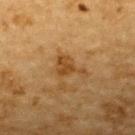The lesion was photographed on a routine skin check and not biopsied; there is no pathology result. The lesion is located on the upper back. The tile uses cross-polarized illumination. A male patient, aged approximately 85 or older. A roughly 15 mm field-of-view crop from a total-body skin photograph.